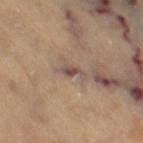- follow-up: catalogued during a skin exam; not biopsied
- site: the right thigh
- lesion size: ≈3.5 mm
- subject: female, aged approximately 70
- lighting: cross-polarized
- acquisition: 15 mm crop, total-body photography